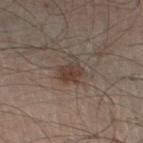This lesion was catalogued during total-body skin photography and was not selected for biopsy. Approximately 3 mm at its widest. Located on the leg. The tile uses white-light illumination. A lesion tile, about 15 mm wide, cut from a 3D total-body photograph. Automated image analysis of the tile measured a lesion area of about 6.5 mm² and two-axis asymmetry of about 0.35. It also reported a lesion color around L≈40 a*≈13 b*≈22 in CIELAB and about 8 CIELAB-L* units darker than the surrounding skin. A male patient in their 60s.